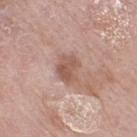notes = catalogued during a skin exam; not biopsied
imaging modality = ~15 mm crop, total-body skin-cancer survey
body site = the right thigh
subject = female, aged 68–72